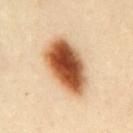Notes:
* follow-up: no biopsy performed (imaged during a skin exam)
* anatomic site: the mid back
* image: ~15 mm crop, total-body skin-cancer survey
* tile lighting: cross-polarized
* subject: female, aged around 40
* automated lesion analysis: a lesion color around L≈55 a*≈24 b*≈38 in CIELAB, about 24 CIELAB-L* units darker than the surrounding skin, and a normalized border contrast of about 14.5
* diameter: ~8 mm (longest diameter)